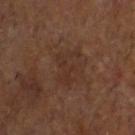Imaged during a routine full-body skin examination; the lesion was not biopsied and no histopathology is available. The total-body-photography lesion software estimated a footprint of about 7.5 mm², an outline eccentricity of about 0.75 (0 = round, 1 = elongated), and two-axis asymmetry of about 0.55. It also reported an average lesion color of about L≈29 a*≈17 b*≈24 (CIELAB), roughly 4 lightness units darker than nearby skin, and a normalized border contrast of about 5. On the head or neck. This image is a 15 mm lesion crop taken from a total-body photograph. The patient is a male aged 58 to 62. Approximately 4.5 mm at its widest. Imaged with cross-polarized lighting.